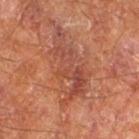Clinical impression:
The lesion was photographed on a routine skin check and not biopsied; there is no pathology result.
Acquisition and patient details:
From the right thigh. The tile uses cross-polarized illumination. The lesion-visualizer software estimated an outline eccentricity of about 0.9 (0 = round, 1 = elongated) and a shape-asymmetry score of about 0.45 (0 = symmetric). It also reported a classifier nevus-likeness of about 0/100 and a lesion-detection confidence of about 75/100. A male subject about 65 years old. A roughly 15 mm field-of-view crop from a total-body skin photograph. Approximately 9 mm at its widest.